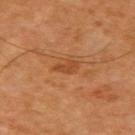Impression: This lesion was catalogued during total-body skin photography and was not selected for biopsy. Clinical summary: Measured at roughly 2.5 mm in maximum diameter. The subject is a male aged around 60. Imaged with cross-polarized lighting. On the right upper arm. A lesion tile, about 15 mm wide, cut from a 3D total-body photograph.